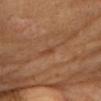Assessment:
This lesion was catalogued during total-body skin photography and was not selected for biopsy.
Background:
From the head or neck. This is a cross-polarized tile. Measured at roughly 1.5 mm in maximum diameter. A close-up tile cropped from a whole-body skin photograph, about 15 mm across. A female patient aged around 65.